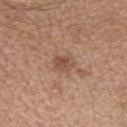A female subject, aged approximately 40.
The lesion is on the head or neck.
A roughly 15 mm field-of-view crop from a total-body skin photograph.